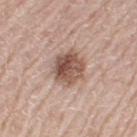workup: imaged on a skin check; not biopsied.